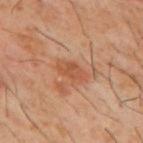Impression: Imaged during a routine full-body skin examination; the lesion was not biopsied and no histopathology is available. Image and clinical context: This image is a 15 mm lesion crop taken from a total-body photograph. A male subject, in their 60s. The lesion is on the mid back. Longest diameter approximately 3.5 mm.